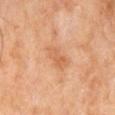Impression: Part of a total-body skin-imaging series; this lesion was reviewed on a skin check and was not flagged for biopsy. Clinical summary: This is a cross-polarized tile. The lesion is located on the abdomen. Cropped from a whole-body photographic skin survey; the tile spans about 15 mm. A male subject approximately 60 years of age.